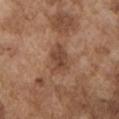Impression: No biopsy was performed on this lesion — it was imaged during a full skin examination and was not determined to be concerning. Clinical summary: A 15 mm crop from a total-body photograph taken for skin-cancer surveillance. Captured under white-light illumination. The lesion is on the left upper arm. A male patient, about 75 years old. Measured at roughly 4 mm in maximum diameter.